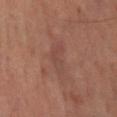<lesion>
<biopsy_status>not biopsied; imaged during a skin examination</biopsy_status>
<automated_metrics>
  <border_irregularity_0_10>5.0</border_irregularity_0_10>
  <peripheral_color_asymmetry>1.0</peripheral_color_asymmetry>
  <nevus_likeness_0_100>0</nevus_likeness_0_100>
  <lesion_detection_confidence_0_100>90</lesion_detection_confidence_0_100>
</automated_metrics>
<patient>
  <sex>male</sex>
  <age_approx>65</age_approx>
</patient>
<lesion_size>
  <long_diameter_mm_approx>4.5</long_diameter_mm_approx>
</lesion_size>
<lighting>cross-polarized</lighting>
<image>
  <source>total-body photography crop</source>
  <field_of_view_mm>15</field_of_view_mm>
</image>
</lesion>